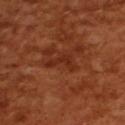Findings:
• workup: catalogued during a skin exam; not biopsied
• body site: the upper back
• patient: female, about 55 years old
• image: ~15 mm crop, total-body skin-cancer survey
• tile lighting: cross-polarized illumination
• size: ≈4 mm
• image-analysis metrics: a footprint of about 4.5 mm² and an eccentricity of roughly 0.9; an average lesion color of about L≈31 a*≈29 b*≈33 (CIELAB) and a normalized lesion–skin contrast near 6.5; an automated nevus-likeness rating near 0 out of 100